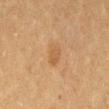Impression: Part of a total-body skin-imaging series; this lesion was reviewed on a skin check and was not flagged for biopsy. Context: On the right thigh. Automated tile analysis of the lesion measured an area of roughly 4.5 mm² and two-axis asymmetry of about 0.2. A female patient, about 55 years old. Cropped from a whole-body photographic skin survey; the tile spans about 15 mm.